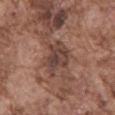Assessment: The lesion was tiled from a total-body skin photograph and was not biopsied. Acquisition and patient details: A region of skin cropped from a whole-body photographic capture, roughly 15 mm wide. The lesion is on the back. An algorithmic analysis of the crop reported a border-irregularity rating of about 3.5/10, internal color variation of about 5 on a 0–10 scale, and peripheral color asymmetry of about 1.5. And it measured lesion-presence confidence of about 90/100. Imaged with white-light lighting. The patient is a male aged 73–77. Approximately 4.5 mm at its widest.